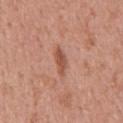Approximately 4 mm at its widest.
The total-body-photography lesion software estimated a mean CIELAB color near L≈53 a*≈25 b*≈30, a lesion–skin lightness drop of about 10, and a normalized lesion–skin contrast near 7. The analysis additionally found a border-irregularity rating of about 3.5/10 and peripheral color asymmetry of about 0.5. The software also gave a classifier nevus-likeness of about 0/100.
A female patient, approximately 50 years of age.
The tile uses white-light illumination.
The lesion is on the upper back.
A 15 mm close-up tile from a total-body photography series done for melanoma screening.